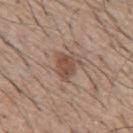Assessment:
Imaged during a routine full-body skin examination; the lesion was not biopsied and no histopathology is available.
Clinical summary:
This image is a 15 mm lesion crop taken from a total-body photograph. The lesion is on the mid back. The subject is a male about 65 years old.